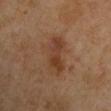Impression:
The lesion was tiled from a total-body skin photograph and was not biopsied.
Acquisition and patient details:
A male subject, in their mid-50s. The lesion is located on the right upper arm. This image is a 15 mm lesion crop taken from a total-body photograph.